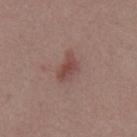Recorded during total-body skin imaging; not selected for excision or biopsy. About 3.5 mm across. The lesion is located on the mid back. Cropped from a whole-body photographic skin survey; the tile spans about 15 mm. Automated tile analysis of the lesion measured a footprint of about 5.5 mm², a shape eccentricity near 0.75, and a shape-asymmetry score of about 0.35 (0 = symmetric). And it measured an average lesion color of about L≈46 a*≈21 b*≈22 (CIELAB), about 9 CIELAB-L* units darker than the surrounding skin, and a normalized lesion–skin contrast near 7. A male subject, in their mid-50s. This is a white-light tile.